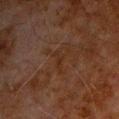Clinical impression:
The lesion was tiled from a total-body skin photograph and was not biopsied.
Context:
A region of skin cropped from a whole-body photographic capture, roughly 15 mm wide. The lesion is located on the chest. Imaged with cross-polarized lighting. Approximately 2.5 mm at its widest. The lesion-visualizer software estimated a lesion color around L≈21 a*≈16 b*≈22 in CIELAB and roughly 3 lightness units darker than nearby skin. A male subject, aged approximately 80.